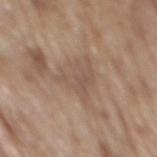Recorded during total-body skin imaging; not selected for excision or biopsy.
Captured under white-light illumination.
A 15 mm close-up tile from a total-body photography series done for melanoma screening.
The recorded lesion diameter is about 5 mm.
The lesion is on the mid back.
A male patient, about 70 years old.